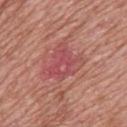The lesion was tiled from a total-body skin photograph and was not biopsied.
Imaged with white-light lighting.
On the upper back.
A male patient, aged around 50.
An algorithmic analysis of the crop reported an average lesion color of about L≈51 a*≈31 b*≈23 (CIELAB), a lesion–skin lightness drop of about 7, and a normalized lesion–skin contrast near 6. It also reported border irregularity of about 5 on a 0–10 scale, a color-variation rating of about 6/10, and peripheral color asymmetry of about 2.5.
Longest diameter approximately 4.5 mm.
A roughly 15 mm field-of-view crop from a total-body skin photograph.